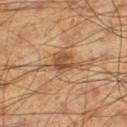No biopsy was performed on this lesion — it was imaged during a full skin examination and was not determined to be concerning. Automated tile analysis of the lesion measured internal color variation of about 4 on a 0–10 scale and radial color variation of about 1.5. About 6 mm across. From the left thigh. The patient is a male approximately 60 years of age. A lesion tile, about 15 mm wide, cut from a 3D total-body photograph.